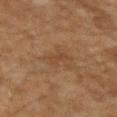Findings:
- notes — no biopsy performed (imaged during a skin exam)
- location — the left upper arm
- image — ~15 mm tile from a whole-body skin photo
- subject — male, approximately 45 years of age